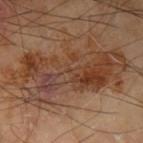Case summary:
* workup — no biopsy performed (imaged during a skin exam)
* anatomic site — the left upper arm
* patient — male, about 65 years old
* image source — ~15 mm crop, total-body skin-cancer survey